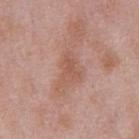Clinical summary: Approximately 3.5 mm at its widest. A male patient, roughly 55 years of age. From the chest. This image is a 15 mm lesion crop taken from a total-body photograph.